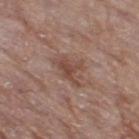This lesion was catalogued during total-body skin photography and was not selected for biopsy.
A 15 mm crop from a total-body photograph taken for skin-cancer surveillance.
From the right thigh.
The tile uses white-light illumination.
About 4.5 mm across.
Automated tile analysis of the lesion measured a shape eccentricity near 0.8 and two-axis asymmetry of about 0.4. The software also gave an average lesion color of about L≈47 a*≈19 b*≈25 (CIELAB), roughly 8 lightness units darker than nearby skin, and a normalized border contrast of about 6.5.
A female subject, roughly 70 years of age.